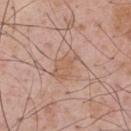This lesion was catalogued during total-body skin photography and was not selected for biopsy. A region of skin cropped from a whole-body photographic capture, roughly 15 mm wide. The tile uses white-light illumination. About 4 mm across. An algorithmic analysis of the crop reported an area of roughly 7 mm² and an eccentricity of roughly 0.85. The analysis additionally found an average lesion color of about L≈58 a*≈20 b*≈29 (CIELAB), about 7 CIELAB-L* units darker than the surrounding skin, and a normalized border contrast of about 5. The software also gave a border-irregularity index near 3.5/10, a color-variation rating of about 2/10, and a peripheral color-asymmetry measure near 0.5. It also reported an automated nevus-likeness rating near 0 out of 100 and a detector confidence of about 100 out of 100 that the crop contains a lesion. The lesion is located on the upper back. A male patient, aged approximately 55.